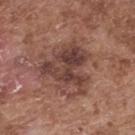Findings:
• biopsy status · total-body-photography surveillance lesion; no biopsy
• diameter · ≈6 mm
• anatomic site · the upper back
• patient · male, in their mid-70s
• imaging modality · 15 mm crop, total-body photography
• lighting · white-light
• automated lesion analysis · an eccentricity of roughly 0.45 and a symmetry-axis asymmetry near 0.5; an average lesion color of about L≈41 a*≈20 b*≈23 (CIELAB) and a lesion-to-skin contrast of about 8.5 (normalized; higher = more distinct); a classifier nevus-likeness of about 15/100